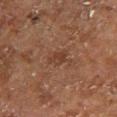workup: catalogued during a skin exam; not biopsied | patient: male, aged around 80 | tile lighting: cross-polarized | imaging modality: ~15 mm tile from a whole-body skin photo | anatomic site: the right lower leg.